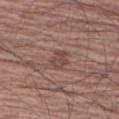| field | value |
|---|---|
| follow-up | catalogued during a skin exam; not biopsied |
| diameter | about 2.5 mm |
| patient | male, approximately 65 years of age |
| illumination | white-light illumination |
| acquisition | ~15 mm crop, total-body skin-cancer survey |
| image-analysis metrics | a mean CIELAB color near L≈46 a*≈19 b*≈23, roughly 8 lightness units darker than nearby skin, and a normalized border contrast of about 6; a within-lesion color-variation index near 2/10 and a peripheral color-asymmetry measure near 1; a detector confidence of about 100 out of 100 that the crop contains a lesion |
| anatomic site | the right upper arm |